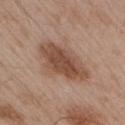notes — catalogued during a skin exam; not biopsied | body site — the arm | tile lighting — white-light | lesion size — ≈6.5 mm | patient — male, roughly 55 years of age | imaging modality — ~15 mm crop, total-body skin-cancer survey.